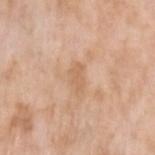follow-up = catalogued during a skin exam; not biopsied
subject = female, aged 73 to 77
anatomic site = the left upper arm
lighting = white-light
image source = ~15 mm crop, total-body skin-cancer survey
lesion size = ≈3 mm